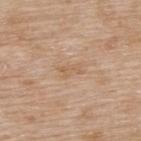| feature | finding |
|---|---|
| workup | imaged on a skin check; not biopsied |
| image source | total-body-photography crop, ~15 mm field of view |
| subject | female, in their mid-70s |
| lighting | white-light |
| automated metrics | an area of roughly 3 mm² and a shape-asymmetry score of about 0.45 (0 = symmetric); border irregularity of about 5.5 on a 0–10 scale, internal color variation of about 0 on a 0–10 scale, and peripheral color asymmetry of about 0 |
| lesion size | ≈3 mm |
| site | the upper back |